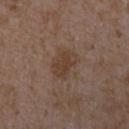{"biopsy_status": "not biopsied; imaged during a skin examination", "lesion_size": {"long_diameter_mm_approx": 3.5}, "site": "right forearm", "patient": {"sex": "female", "age_approx": 35}, "lighting": "white-light", "image": {"source": "total-body photography crop", "field_of_view_mm": 15}}